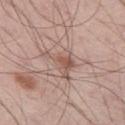automated metrics: an eccentricity of roughly 0.75 and a shape-asymmetry score of about 0.6 (0 = symmetric); border irregularity of about 7 on a 0–10 scale, a color-variation rating of about 5/10, and peripheral color asymmetry of about 2 | body site: the left thigh | patient: male, roughly 60 years of age | acquisition: ~15 mm tile from a whole-body skin photo.